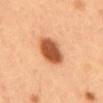• automated lesion analysis: a lesion area of about 12 mm² and an outline eccentricity of about 0.65 (0 = round, 1 = elongated); an average lesion color of about L≈56 a*≈28 b*≈40 (CIELAB) and a normalized border contrast of about 11.5; a border-irregularity index near 1.5/10, a color-variation rating of about 4.5/10, and a peripheral color-asymmetry measure near 1.5; lesion-presence confidence of about 100/100
• lesion size: about 4.5 mm
• patient: male, aged around 50
• imaging modality: 15 mm crop, total-body photography
• tile lighting: cross-polarized
• site: the abdomen